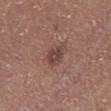The lesion was tiled from a total-body skin photograph and was not biopsied. The patient is a female roughly 50 years of age. The lesion's longest dimension is about 2.5 mm. A 15 mm close-up tile from a total-body photography series done for melanoma screening. The lesion is located on the right lower leg. This is a white-light tile.